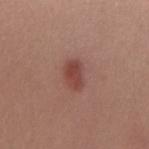The lesion was photographed on a routine skin check and not biopsied; there is no pathology result. The lesion is located on the back. Cropped from a whole-body photographic skin survey; the tile spans about 15 mm. A female subject, about 35 years old.